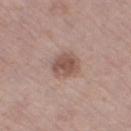No biopsy was performed on this lesion — it was imaged during a full skin examination and was not determined to be concerning.
Captured under white-light illumination.
A 15 mm close-up extracted from a 3D total-body photography capture.
The recorded lesion diameter is about 3.5 mm.
Located on the leg.
The patient is a female aged around 65.